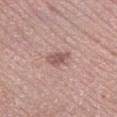Captured during whole-body skin photography for melanoma surveillance; the lesion was not biopsied. The subject is a male aged 18 to 22. On the right lower leg. Cropped from a whole-body photographic skin survey; the tile spans about 15 mm.